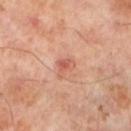Q: Is there a histopathology result?
A: imaged on a skin check; not biopsied
Q: How large is the lesion?
A: ~2.5 mm (longest diameter)
Q: Automated lesion metrics?
A: a footprint of about 3.5 mm², an outline eccentricity of about 0.75 (0 = round, 1 = elongated), and a shape-asymmetry score of about 0.45 (0 = symmetric); a mean CIELAB color near L≈58 a*≈27 b*≈31, about 9 CIELAB-L* units darker than the surrounding skin, and a normalized border contrast of about 6; border irregularity of about 4.5 on a 0–10 scale and a peripheral color-asymmetry measure near 0.5
Q: Where on the body is the lesion?
A: the left lower leg
Q: Who is the patient?
A: male, in their 50s
Q: How was the tile lit?
A: cross-polarized
Q: How was this image acquired?
A: 15 mm crop, total-body photography